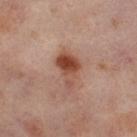biopsy_status: not biopsied; imaged during a skin examination
lesion_size:
  long_diameter_mm_approx: 4.5
patient:
  sex: female
  age_approx: 55
image:
  source: total-body photography crop
  field_of_view_mm: 15
site: left thigh